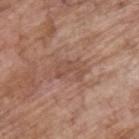Impression:
Part of a total-body skin-imaging series; this lesion was reviewed on a skin check and was not flagged for biopsy.
Acquisition and patient details:
Imaged with white-light lighting. The lesion is located on the upper back. A male subject approximately 70 years of age. Longest diameter approximately 3.5 mm. A roughly 15 mm field-of-view crop from a total-body skin photograph.